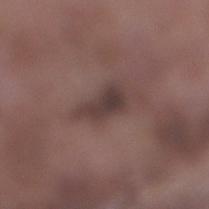Recorded during total-body skin imaging; not selected for excision or biopsy.
The lesion is located on the leg.
The subject is a male in their mid-70s.
A 15 mm close-up tile from a total-body photography series done for melanoma screening.